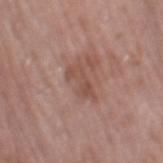Imaged during a routine full-body skin examination; the lesion was not biopsied and no histopathology is available.